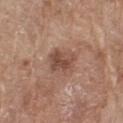Q: Was a biopsy performed?
A: no biopsy performed (imaged during a skin exam)
Q: How was the tile lit?
A: white-light
Q: Who is the patient?
A: female, aged approximately 75
Q: What did automated image analysis measure?
A: an average lesion color of about L≈48 a*≈20 b*≈27 (CIELAB) and roughly 10 lightness units darker than nearby skin; a border-irregularity index near 2/10, a within-lesion color-variation index near 4.5/10, and peripheral color asymmetry of about 1.5; an automated nevus-likeness rating near 15 out of 100 and lesion-presence confidence of about 100/100
Q: Lesion size?
A: ≈4 mm
Q: Lesion location?
A: the right thigh
Q: What kind of image is this?
A: 15 mm crop, total-body photography